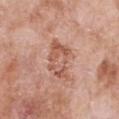follow-up: catalogued during a skin exam; not biopsied
tile lighting: white-light
image: total-body-photography crop, ~15 mm field of view
subject: female, aged around 75
body site: the front of the torso